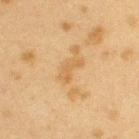Impression: The lesion was tiled from a total-body skin photograph and was not biopsied. Clinical summary: Longest diameter approximately 3.5 mm. A female patient aged around 40. Cropped from a whole-body photographic skin survey; the tile spans about 15 mm. Located on the arm. The tile uses cross-polarized illumination. The total-body-photography lesion software estimated a lesion–skin lightness drop of about 6 and a lesion-to-skin contrast of about 5.5 (normalized; higher = more distinct). It also reported border irregularity of about 5 on a 0–10 scale and radial color variation of about 0.5.